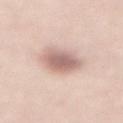follow-up: catalogued during a skin exam; not biopsied
body site: the back
lighting: white-light
diameter: ≈4 mm
imaging modality: ~15 mm crop, total-body skin-cancer survey
patient: male, aged 48 to 52